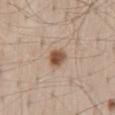This lesion was catalogued during total-body skin photography and was not selected for biopsy.
This is a white-light tile.
A 15 mm close-up extracted from a 3D total-body photography capture.
A male subject aged around 60.
From the lower back.